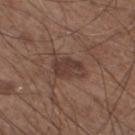| feature | finding |
|---|---|
| biopsy status | imaged on a skin check; not biopsied |
| anatomic site | the left lower leg |
| illumination | white-light |
| acquisition | ~15 mm crop, total-body skin-cancer survey |
| subject | male, roughly 60 years of age |
| lesion diameter | ~4 mm (longest diameter) |